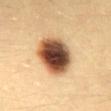Clinical impression: Recorded during total-body skin imaging; not selected for excision or biopsy. Image and clinical context: Located on the mid back. A female patient, approximately 30 years of age. A close-up tile cropped from a whole-body skin photograph, about 15 mm across.